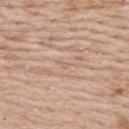Part of a total-body skin-imaging series; this lesion was reviewed on a skin check and was not flagged for biopsy.
From the back.
The tile uses white-light illumination.
The recorded lesion diameter is about 1 mm.
A 15 mm close-up tile from a total-body photography series done for melanoma screening.
The patient is a female aged 48 to 52.